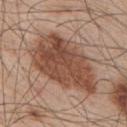A 15 mm close-up tile from a total-body photography series done for melanoma screening.
The tile uses white-light illumination.
On the upper back.
Measured at roughly 9.5 mm in maximum diameter.
A male subject about 55 years old.